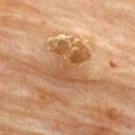Notes:
• follow-up — imaged on a skin check; not biopsied